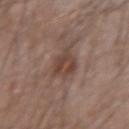Part of a total-body skin-imaging series; this lesion was reviewed on a skin check and was not flagged for biopsy.
The recorded lesion diameter is about 4.5 mm.
Cropped from a whole-body photographic skin survey; the tile spans about 15 mm.
On the left forearm.
Captured under white-light illumination.
A male subject, about 30 years old.